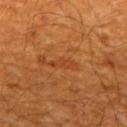| feature | finding |
|---|---|
| notes | no biopsy performed (imaged during a skin exam) |
| lighting | cross-polarized |
| image source | ~15 mm tile from a whole-body skin photo |
| lesion size | ~3.5 mm (longest diameter) |
| subject | male, approximately 60 years of age |
| location | the mid back |